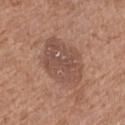Clinical impression: The lesion was photographed on a routine skin check and not biopsied; there is no pathology result. Clinical summary: The subject is a female in their mid-70s. From the leg. A lesion tile, about 15 mm wide, cut from a 3D total-body photograph.